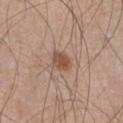Q: What kind of image is this?
A: 15 mm crop, total-body photography
Q: How large is the lesion?
A: ~2.5 mm (longest diameter)
Q: Patient demographics?
A: male, in their mid-40s
Q: Where on the body is the lesion?
A: the left lower leg